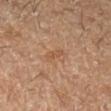Impression:
No biopsy was performed on this lesion — it was imaged during a full skin examination and was not determined to be concerning.
Context:
This image is a 15 mm lesion crop taken from a total-body photograph. Automated image analysis of the tile measured an eccentricity of roughly 0.8. And it measured a border-irregularity index near 3/10, a color-variation rating of about 2.5/10, and a peripheral color-asymmetry measure near 1. And it measured an automated nevus-likeness rating near 0 out of 100 and a lesion-detection confidence of about 100/100. From the left forearm. A male patient aged 53 to 57.